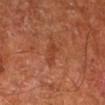Findings:
* follow-up · no biopsy performed (imaged during a skin exam)
* illumination · cross-polarized illumination
* anatomic site · the leg
* image · ~15 mm tile from a whole-body skin photo
* subject · male, about 65 years old
* diameter · ~2.5 mm (longest diameter)